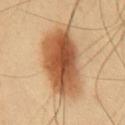The lesion is on the chest.
A male subject aged 38 to 42.
Cropped from a total-body skin-imaging series; the visible field is about 15 mm.